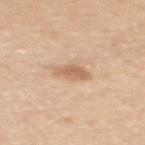Captured during whole-body skin photography for melanoma surveillance; the lesion was not biopsied. The lesion is located on the back. Approximately 3 mm at its widest. A roughly 15 mm field-of-view crop from a total-body skin photograph. The patient is a female aged 43–47.